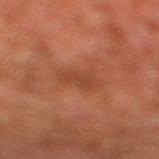Case summary:
* follow-up: total-body-photography surveillance lesion; no biopsy
* TBP lesion metrics: a lesion area of about 6 mm², an outline eccentricity of about 0.7 (0 = round, 1 = elongated), and a symmetry-axis asymmetry near 0.3; a lesion color around L≈34 a*≈21 b*≈27 in CIELAB, roughly 5 lightness units darker than nearby skin, and a lesion-to-skin contrast of about 5 (normalized; higher = more distinct); a border-irregularity rating of about 4/10 and a within-lesion color-variation index near 1.5/10
* image source: ~15 mm tile from a whole-body skin photo
* lesion diameter: ≈3 mm
* subject: male, aged approximately 80
* location: the right lower leg